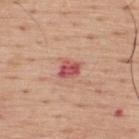lesion size=about 3 mm
tile lighting=white-light
subject=male, roughly 55 years of age
site=the upper back
imaging modality=total-body-photography crop, ~15 mm field of view